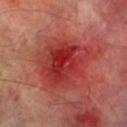biopsy_status: not biopsied; imaged during a skin examination
site: right lower leg
image:
  source: total-body photography crop
  field_of_view_mm: 15
patient:
  sex: male
  age_approx: 70